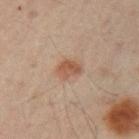Part of a total-body skin-imaging series; this lesion was reviewed on a skin check and was not flagged for biopsy.
Measured at roughly 3 mm in maximum diameter.
A male patient about 50 years old.
A lesion tile, about 15 mm wide, cut from a 3D total-body photograph.
The total-body-photography lesion software estimated a footprint of about 5 mm², an outline eccentricity of about 0.55 (0 = round, 1 = elongated), and a symmetry-axis asymmetry near 0.25. The analysis additionally found roughly 8 lightness units darker than nearby skin and a normalized lesion–skin contrast near 7. The software also gave a border-irregularity rating of about 2.5/10, a within-lesion color-variation index near 3/10, and peripheral color asymmetry of about 1. The analysis additionally found a classifier nevus-likeness of about 90/100 and a detector confidence of about 100 out of 100 that the crop contains a lesion.
From the left upper arm.